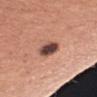Imaged during a routine full-body skin examination; the lesion was not biopsied and no histopathology is available. A 15 mm crop from a total-body photograph taken for skin-cancer surveillance. The patient is a female aged 53 to 57. Located on the left forearm. The total-body-photography lesion software estimated a footprint of about 6 mm² and two-axis asymmetry of about 0.2. And it measured an average lesion color of about L≈46 a*≈21 b*≈24 (CIELAB) and roughly 19 lightness units darker than nearby skin. The recorded lesion diameter is about 3.5 mm. Imaged with white-light lighting.